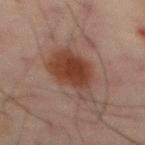The lesion was photographed on a routine skin check and not biopsied; there is no pathology result. The subject is a male in their mid-60s. The recorded lesion diameter is about 6.5 mm. The lesion is located on the back. This is a cross-polarized tile. A 15 mm crop from a total-body photograph taken for skin-cancer surveillance.